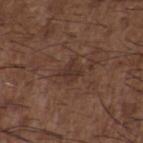Impression: Captured during whole-body skin photography for melanoma surveillance; the lesion was not biopsied. Image and clinical context: The subject is a male about 50 years old. The tile uses white-light illumination. The recorded lesion diameter is about 3 mm. A lesion tile, about 15 mm wide, cut from a 3D total-body photograph. The lesion is on the upper back.